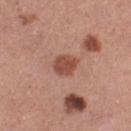This lesion was catalogued during total-body skin photography and was not selected for biopsy. This is a white-light tile. A lesion tile, about 15 mm wide, cut from a 3D total-body photograph. The lesion is on the leg. The lesion-visualizer software estimated an automated nevus-likeness rating near 90 out of 100 and a detector confidence of about 100 out of 100 that the crop contains a lesion. The subject is a female about 30 years old. Measured at roughly 3 mm in maximum diameter.